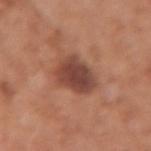Recorded during total-body skin imaging; not selected for excision or biopsy. A female subject, aged approximately 50. The recorded lesion diameter is about 4.5 mm. A 15 mm close-up tile from a total-body photography series done for melanoma screening. Located on the left forearm.